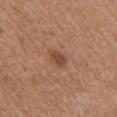Assessment: Captured during whole-body skin photography for melanoma surveillance; the lesion was not biopsied. Context: On the arm. Approximately 3 mm at its widest. Automated image analysis of the tile measured a border-irregularity rating of about 2.5/10, a within-lesion color-variation index near 3/10, and a peripheral color-asymmetry measure near 1. A female subject, aged approximately 55. A 15 mm crop from a total-body photograph taken for skin-cancer surveillance.